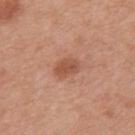Q: What is the imaging modality?
A: ~15 mm crop, total-body skin-cancer survey
Q: How large is the lesion?
A: about 3 mm
Q: What did automated image analysis measure?
A: an area of roughly 5 mm², a shape eccentricity near 0.75, and a symmetry-axis asymmetry near 0.25; a lesion-detection confidence of about 100/100
Q: What lighting was used for the tile?
A: white-light illumination
Q: Patient demographics?
A: male, aged approximately 70
Q: What is the anatomic site?
A: the mid back